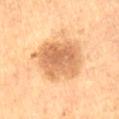notes — imaged on a skin check; not biopsied
tile lighting — cross-polarized
image source — total-body-photography crop, ~15 mm field of view
automated metrics — a mean CIELAB color near L≈65 a*≈22 b*≈40, a lesion–skin lightness drop of about 13, and a lesion-to-skin contrast of about 8 (normalized; higher = more distinct); a classifier nevus-likeness of about 15/100
anatomic site — the right thigh
subject — female, approximately 70 years of age